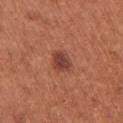Case summary:
* workup · no biopsy performed (imaged during a skin exam)
* subject · female, approximately 65 years of age
* acquisition · ~15 mm tile from a whole-body skin photo
* site · the arm
* lesion size · ≈2.5 mm
* lighting · white-light
* automated lesion analysis · a footprint of about 5.5 mm² and an eccentricity of roughly 0.5; a border-irregularity index near 1/10, a within-lesion color-variation index near 3.5/10, and peripheral color asymmetry of about 1; a nevus-likeness score of about 90/100 and lesion-presence confidence of about 100/100